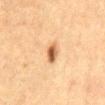biopsy status: no biopsy performed (imaged during a skin exam) | location: the abdomen | patient: female, approximately 60 years of age | size: about 3 mm | imaging modality: ~15 mm tile from a whole-body skin photo | lighting: cross-polarized.